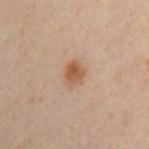Clinical impression:
The lesion was photographed on a routine skin check and not biopsied; there is no pathology result.
Context:
A 15 mm close-up extracted from a 3D total-body photography capture. Imaged with cross-polarized lighting. From the left upper arm. A male patient, aged around 35. Longest diameter approximately 3 mm.